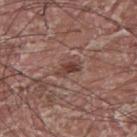Assessment: This lesion was catalogued during total-body skin photography and was not selected for biopsy. Clinical summary: About 3 mm across. A male subject, approximately 40 years of age. Cropped from a total-body skin-imaging series; the visible field is about 15 mm. The lesion is located on the back. The tile uses white-light illumination.